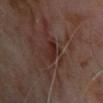Findings:
• follow-up · catalogued during a skin exam; not biopsied
• location · the chest
• image · total-body-photography crop, ~15 mm field of view
• subject · male, aged approximately 65
• lighting · cross-polarized